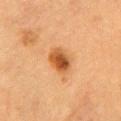The lesion was tiled from a total-body skin photograph and was not biopsied. A 15 mm crop from a total-body photograph taken for skin-cancer surveillance. The tile uses cross-polarized illumination. The lesion is on the chest. The lesion-visualizer software estimated an outline eccentricity of about 0.75 (0 = round, 1 = elongated) and a symmetry-axis asymmetry near 0.15. The analysis additionally found a border-irregularity rating of about 1.5/10. And it measured a nevus-likeness score of about 100/100 and lesion-presence confidence of about 100/100. A male subject roughly 85 years of age or older.